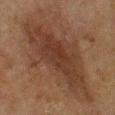No biopsy was performed on this lesion — it was imaged during a full skin examination and was not determined to be concerning. Cropped from a total-body skin-imaging series; the visible field is about 15 mm. A male patient aged approximately 60. Measured at roughly 13 mm in maximum diameter. The lesion is on the leg.